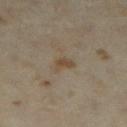The lesion was photographed on a routine skin check and not biopsied; there is no pathology result. This is a cross-polarized tile. The subject is a female aged approximately 35. Automated tile analysis of the lesion measured a border-irregularity index near 3.5/10, a within-lesion color-variation index near 1.5/10, and radial color variation of about 0.5. The software also gave an automated nevus-likeness rating near 10 out of 100 and lesion-presence confidence of about 100/100. The lesion's longest dimension is about 2.5 mm. A lesion tile, about 15 mm wide, cut from a 3D total-body photograph. Located on the right lower leg.